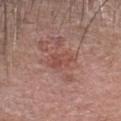Q: Was this lesion biopsied?
A: no biopsy performed (imaged during a skin exam)
Q: How was this image acquired?
A: total-body-photography crop, ~15 mm field of view
Q: How was the tile lit?
A: white-light illumination
Q: What did automated image analysis measure?
A: border irregularity of about 4 on a 0–10 scale, internal color variation of about 2.5 on a 0–10 scale, and peripheral color asymmetry of about 1
Q: Who is the patient?
A: male, in their mid-60s
Q: Where on the body is the lesion?
A: the head or neck
Q: How large is the lesion?
A: ≈3 mm